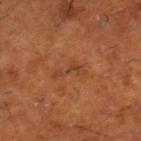– workup: imaged on a skin check; not biopsied
– imaging modality: ~15 mm tile from a whole-body skin photo
– patient: male, about 65 years old
– location: the right lower leg
– size: ~4 mm (longest diameter)